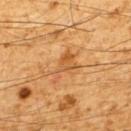The lesion was photographed on a routine skin check and not biopsied; there is no pathology result. A male subject, aged around 60. On the upper back. About 4.5 mm across. Automated image analysis of the tile measured an area of roughly 6 mm², an outline eccentricity of about 0.85 (0 = round, 1 = elongated), and two-axis asymmetry of about 0.5. The software also gave a classifier nevus-likeness of about 5/100. Captured under cross-polarized illumination. Cropped from a total-body skin-imaging series; the visible field is about 15 mm.